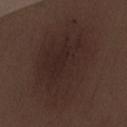No biopsy was performed on this lesion — it was imaged during a full skin examination and was not determined to be concerning. A region of skin cropped from a whole-body photographic capture, roughly 15 mm wide. From the chest. This is a white-light tile. A male patient, roughly 30 years of age.